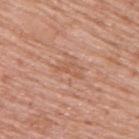Q: Was a biopsy performed?
A: imaged on a skin check; not biopsied
Q: What is the imaging modality?
A: 15 mm crop, total-body photography
Q: Patient demographics?
A: male, approximately 70 years of age
Q: What is the anatomic site?
A: the upper back